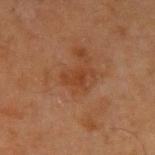biopsy status=catalogued during a skin exam; not biopsied | size=about 3.5 mm | tile lighting=cross-polarized | body site=the left upper arm | acquisition=~15 mm tile from a whole-body skin photo | automated lesion analysis=border irregularity of about 4.5 on a 0–10 scale and a within-lesion color-variation index near 2.5/10 | subject=male, roughly 65 years of age.